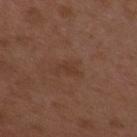workup: total-body-photography surveillance lesion; no biopsy | lighting: white-light | patient: female, aged 33 to 37 | automated lesion analysis: an average lesion color of about L≈36 a*≈19 b*≈27 (CIELAB); border irregularity of about 4.5 on a 0–10 scale, internal color variation of about 0 on a 0–10 scale, and a peripheral color-asymmetry measure near 0; a detector confidence of about 100 out of 100 that the crop contains a lesion | image: ~15 mm tile from a whole-body skin photo | body site: the chest | lesion size: about 2.5 mm.